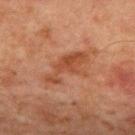<case>
  <image>
    <source>total-body photography crop</source>
    <field_of_view_mm>15</field_of_view_mm>
  </image>
  <lighting>cross-polarized</lighting>
  <site>chest</site>
  <patient>
    <sex>male</sex>
    <age_approx>65</age_approx>
  </patient>
  <lesion_size>
    <long_diameter_mm_approx>5.5</long_diameter_mm_approx>
  </lesion_size>
  <automated_metrics>
    <area_mm2_approx>10.0</area_mm2_approx>
    <eccentricity>0.9</eccentricity>
    <shape_asymmetry>0.5</shape_asymmetry>
    <cielab_L>38</cielab_L>
    <cielab_a>22</cielab_a>
    <cielab_b>29</cielab_b>
    <vs_skin_darker_L>7.0</vs_skin_darker_L>
    <color_variation_0_10>2.5</color_variation_0_10>
    <peripheral_color_asymmetry>1.0</peripheral_color_asymmetry>
    <lesion_detection_confidence_0_100>100</lesion_detection_confidence_0_100>
  </automated_metrics>
</case>